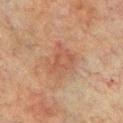Imaged during a routine full-body skin examination; the lesion was not biopsied and no histopathology is available.
Located on the chest.
This is a cross-polarized tile.
A male subject aged 73 to 77.
The lesion's longest dimension is about 5 mm.
This image is a 15 mm lesion crop taken from a total-body photograph.